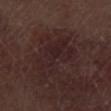Clinical impression:
Recorded during total-body skin imaging; not selected for excision or biopsy.
Background:
Automated image analysis of the tile measured a border-irregularity rating of about 5.5/10, a color-variation rating of about 3.5/10, and radial color variation of about 1. A male patient, aged 68–72. Located on the left thigh. Measured at roughly 6.5 mm in maximum diameter. Cropped from a whole-body photographic skin survey; the tile spans about 15 mm.